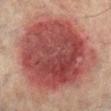Q: How was this image acquired?
A: ~15 mm tile from a whole-body skin photo
Q: Where on the body is the lesion?
A: the left lower leg
Q: Who is the patient?
A: male, approximately 75 years of age